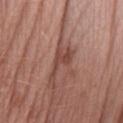Q: Was a biopsy performed?
A: no biopsy performed (imaged during a skin exam)
Q: How large is the lesion?
A: ≈5 mm
Q: What kind of image is this?
A: ~15 mm tile from a whole-body skin photo
Q: Lesion location?
A: the arm
Q: Automated lesion metrics?
A: a classifier nevus-likeness of about 0/100 and a detector confidence of about 75 out of 100 that the crop contains a lesion
Q: What lighting was used for the tile?
A: white-light illumination
Q: What are the patient's age and sex?
A: female, aged around 60